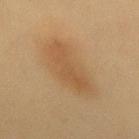The lesion was tiled from a total-body skin photograph and was not biopsied.
Captured under cross-polarized illumination.
Automated image analysis of the tile measured a lesion area of about 22 mm² and two-axis asymmetry of about 0.25. The analysis additionally found a border-irregularity index near 3/10, a within-lesion color-variation index near 3/10, and peripheral color asymmetry of about 1. And it measured a detector confidence of about 100 out of 100 that the crop contains a lesion.
The patient is a female aged 38 to 42.
Located on the mid back.
Cropped from a total-body skin-imaging series; the visible field is about 15 mm.